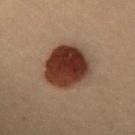biopsy status = total-body-photography surveillance lesion; no biopsy
tile lighting = cross-polarized illumination
subject = female, aged around 30
site = the chest
imaging modality = ~15 mm tile from a whole-body skin photo